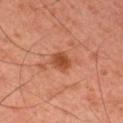Assessment:
Imaged during a routine full-body skin examination; the lesion was not biopsied and no histopathology is available.
Context:
The lesion is located on the right upper arm. A close-up tile cropped from a whole-body skin photograph, about 15 mm across. The subject is a male roughly 45 years of age.